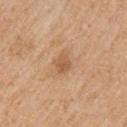Assessment: The lesion was photographed on a routine skin check and not biopsied; there is no pathology result. Background: Captured under white-light illumination. The recorded lesion diameter is about 2.5 mm. A region of skin cropped from a whole-body photographic capture, roughly 15 mm wide. The total-body-photography lesion software estimated a lesion area of about 4.5 mm², a shape eccentricity near 0.65, and two-axis asymmetry of about 0.2. It also reported a lesion color around L≈58 a*≈21 b*≈36 in CIELAB and roughly 9 lightness units darker than nearby skin. A female subject, about 45 years old. Located on the right upper arm.